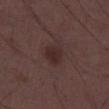Q: Is there a histopathology result?
A: imaged on a skin check; not biopsied
Q: How was this image acquired?
A: ~15 mm crop, total-body skin-cancer survey
Q: What did automated image analysis measure?
A: an average lesion color of about L≈26 a*≈17 b*≈17 (CIELAB) and a lesion-to-skin contrast of about 7.5 (normalized; higher = more distinct); a border-irregularity index near 2/10, internal color variation of about 1.5 on a 0–10 scale, and peripheral color asymmetry of about 0.5; a detector confidence of about 100 out of 100 that the crop contains a lesion
Q: What is the lesion's diameter?
A: about 2.5 mm
Q: How was the tile lit?
A: white-light
Q: Patient demographics?
A: male, in their 50s